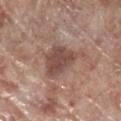Impression: This lesion was catalogued during total-body skin photography and was not selected for biopsy. Background: The patient is a male aged 68 to 72. A close-up tile cropped from a whole-body skin photograph, about 15 mm across. About 5.5 mm across. An algorithmic analysis of the crop reported an area of roughly 13 mm², an outline eccentricity of about 0.7 (0 = round, 1 = elongated), and two-axis asymmetry of about 0.4. The software also gave a lesion color around L≈48 a*≈19 b*≈23 in CIELAB and a lesion-to-skin contrast of about 8 (normalized; higher = more distinct). Imaged with white-light lighting. On the right lower leg.